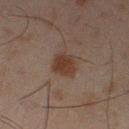Case summary:
– workup — imaged on a skin check; not biopsied
– lesion size — about 2.5 mm
– automated lesion analysis — a lesion area of about 6 mm², an eccentricity of roughly 0.35, and a symmetry-axis asymmetry near 0.15; internal color variation of about 2 on a 0–10 scale and a peripheral color-asymmetry measure near 0.5; an automated nevus-likeness rating near 95 out of 100 and a lesion-detection confidence of about 100/100
– subject — male, in their mid- to late 40s
– image — ~15 mm crop, total-body skin-cancer survey
– lighting — cross-polarized illumination
– location — the left lower leg